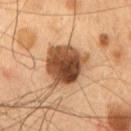Q: Was a biopsy performed?
A: imaged on a skin check; not biopsied
Q: What is the imaging modality?
A: ~15 mm crop, total-body skin-cancer survey
Q: What is the anatomic site?
A: the chest
Q: What lighting was used for the tile?
A: cross-polarized illumination
Q: Who is the patient?
A: male, in their mid-50s
Q: Automated lesion metrics?
A: a footprint of about 18 mm², an eccentricity of roughly 0.35, and a shape-asymmetry score of about 0.2 (0 = symmetric); a mean CIELAB color near L≈43 a*≈21 b*≈32 and a lesion-to-skin contrast of about 13.5 (normalized; higher = more distinct); a border-irregularity rating of about 2.5/10 and a color-variation rating of about 8/10